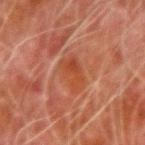Clinical impression: Recorded during total-body skin imaging; not selected for excision or biopsy. Clinical summary: A male patient aged 78 to 82. This is a cross-polarized tile. The lesion is on the left forearm. A close-up tile cropped from a whole-body skin photograph, about 15 mm across.